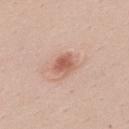Assessment:
This lesion was catalogued during total-body skin photography and was not selected for biopsy.
Context:
The lesion is located on the upper back. Cropped from a whole-body photographic skin survey; the tile spans about 15 mm. The patient is a male aged around 50.